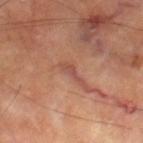Captured during whole-body skin photography for melanoma surveillance; the lesion was not biopsied. The tile uses cross-polarized illumination. Measured at roughly 3 mm in maximum diameter. A male subject, aged around 70. This image is a 15 mm lesion crop taken from a total-body photograph. From the left thigh.